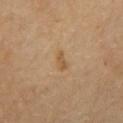The lesion's longest dimension is about 2.5 mm.
The patient is a male roughly 70 years of age.
Captured under cross-polarized illumination.
This image is a 15 mm lesion crop taken from a total-body photograph.
The total-body-photography lesion software estimated two-axis asymmetry of about 0.35. The software also gave roughly 7 lightness units darker than nearby skin and a lesion-to-skin contrast of about 6 (normalized; higher = more distinct). It also reported border irregularity of about 3.5 on a 0–10 scale, a color-variation rating of about 1/10, and a peripheral color-asymmetry measure near 0.5. And it measured an automated nevus-likeness rating near 5 out of 100 and a detector confidence of about 100 out of 100 that the crop contains a lesion.
The lesion is on the chest.